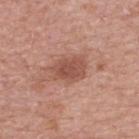This lesion was catalogued during total-body skin photography and was not selected for biopsy. Located on the upper back. This is a white-light tile. The patient is a female aged 58 to 62. A region of skin cropped from a whole-body photographic capture, roughly 15 mm wide.